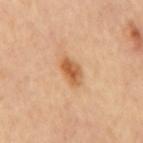biopsy status = catalogued during a skin exam; not biopsied
lesion size = ≈3.5 mm
location = the back
automated lesion analysis = an average lesion color of about L≈60 a*≈24 b*≈41 (CIELAB), roughly 13 lightness units darker than nearby skin, and a normalized border contrast of about 8.5
acquisition = total-body-photography crop, ~15 mm field of view
lighting = cross-polarized illumination